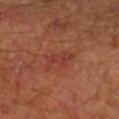biopsy status: total-body-photography surveillance lesion; no biopsy | image-analysis metrics: an average lesion color of about L≈38 a*≈28 b*≈29 (CIELAB), a lesion–skin lightness drop of about 6, and a normalized lesion–skin contrast near 5; border irregularity of about 6 on a 0–10 scale, a within-lesion color-variation index near 3/10, and radial color variation of about 1; an automated nevus-likeness rating near 5 out of 100 and a detector confidence of about 100 out of 100 that the crop contains a lesion | patient: male, aged 53–57 | site: the arm | imaging modality: ~15 mm crop, total-body skin-cancer survey.